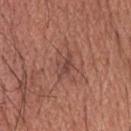workup=catalogued during a skin exam; not biopsied | image=~15 mm tile from a whole-body skin photo | location=the head or neck | subject=male, roughly 55 years of age | lighting=white-light | lesion diameter=≈3 mm | image-analysis metrics=a border-irregularity rating of about 4.5/10, a color-variation rating of about 2.5/10, and peripheral color asymmetry of about 1.